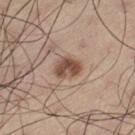<record>
<biopsy_status>not biopsied; imaged during a skin examination</biopsy_status>
<lighting>white-light</lighting>
<site>left thigh</site>
<lesion_size>
  <long_diameter_mm_approx>3.5</long_diameter_mm_approx>
</lesion_size>
<patient>
  <sex>male</sex>
  <age_approx>55</age_approx>
</patient>
<automated_metrics>
  <area_mm2_approx>6.5</area_mm2_approx>
  <lesion_detection_confidence_0_100>100</lesion_detection_confidence_0_100>
</automated_metrics>
<image>
  <source>total-body photography crop</source>
  <field_of_view_mm>15</field_of_view_mm>
</image>
</record>